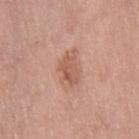Impression:
Recorded during total-body skin imaging; not selected for excision or biopsy.
Context:
Approximately 4.5 mm at its widest. Captured under white-light illumination. A female subject, aged 63–67. On the right thigh. A close-up tile cropped from a whole-body skin photograph, about 15 mm across.